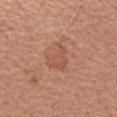The lesion was tiled from a total-body skin photograph and was not biopsied. A female patient about 35 years old. Automated image analysis of the tile measured a lesion area of about 6 mm², an outline eccentricity of about 0.65 (0 = round, 1 = elongated), and a symmetry-axis asymmetry near 0.55. And it measured a classifier nevus-likeness of about 0/100 and lesion-presence confidence of about 100/100. Longest diameter approximately 3.5 mm. This image is a 15 mm lesion crop taken from a total-body photograph. Located on the left forearm. Imaged with white-light lighting.